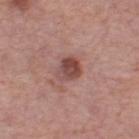Part of a total-body skin-imaging series; this lesion was reviewed on a skin check and was not flagged for biopsy. The recorded lesion diameter is about 3 mm. The lesion is on the left thigh. This image is a 15 mm lesion crop taken from a total-body photograph. Captured under white-light illumination. A female subject aged around 55. Automated image analysis of the tile measured a lesion color around L≈46 a*≈23 b*≈23 in CIELAB and a normalized border contrast of about 8.5. And it measured internal color variation of about 5 on a 0–10 scale and a peripheral color-asymmetry measure near 2.